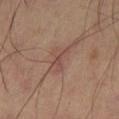• notes — imaged on a skin check; not biopsied
• location — the leg
• lighting — cross-polarized illumination
• imaging modality — total-body-photography crop, ~15 mm field of view
• lesion diameter — about 3.5 mm